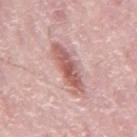biopsy_status: not biopsied; imaged during a skin examination
site: mid back
image:
  source: total-body photography crop
  field_of_view_mm: 15
lesion_size:
  long_diameter_mm_approx: 6.0
patient:
  sex: male
  age_approx: 75
lighting: white-light